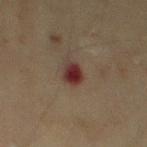<case>
<biopsy_status>not biopsied; imaged during a skin examination</biopsy_status>
<image>
  <source>total-body photography crop</source>
  <field_of_view_mm>15</field_of_view_mm>
</image>
<lesion_size>
  <long_diameter_mm_approx>2.5</long_diameter_mm_approx>
</lesion_size>
<lighting>cross-polarized</lighting>
<site>left thigh</site>
<patient>
  <sex>female</sex>
  <age_approx>55</age_approx>
</patient>
</case>